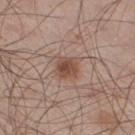Q: What lighting was used for the tile?
A: white-light illumination
Q: Where on the body is the lesion?
A: the leg
Q: How was this image acquired?
A: ~15 mm tile from a whole-body skin photo
Q: What did automated image analysis measure?
A: an average lesion color of about L≈48 a*≈20 b*≈27 (CIELAB) and roughly 10 lightness units darker than nearby skin; border irregularity of about 1.5 on a 0–10 scale, internal color variation of about 3.5 on a 0–10 scale, and peripheral color asymmetry of about 1.5; a nevus-likeness score of about 90/100
Q: What are the patient's age and sex?
A: male, aged approximately 60